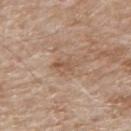Assessment: No biopsy was performed on this lesion — it was imaged during a full skin examination and was not determined to be concerning. Background: This is a white-light tile. The patient is a male aged approximately 80. On the back. About 3 mm across. This image is a 15 mm lesion crop taken from a total-body photograph.